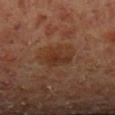Notes:
• notes · no biopsy performed (imaged during a skin exam)
• illumination · cross-polarized
• location · the left lower leg
• imaging modality · total-body-photography crop, ~15 mm field of view
• patient · male, aged around 60